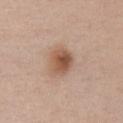Q: Was a biopsy performed?
A: total-body-photography surveillance lesion; no biopsy
Q: What is the anatomic site?
A: the chest
Q: What kind of image is this?
A: 15 mm crop, total-body photography
Q: What did automated image analysis measure?
A: a mean CIELAB color near L≈54 a*≈19 b*≈30 and a lesion–skin lightness drop of about 12
Q: Who is the patient?
A: female, about 40 years old
Q: Lesion size?
A: ~3.5 mm (longest diameter)